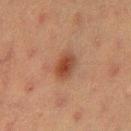Captured during whole-body skin photography for melanoma surveillance; the lesion was not biopsied.